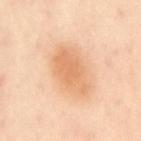Notes:
- workup: imaged on a skin check; not biopsied
- subject: female, in their mid-50s
- acquisition: ~15 mm tile from a whole-body skin photo
- body site: the upper back